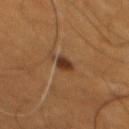Findings:
- workup: no biopsy performed (imaged during a skin exam)
- diameter: about 2.5 mm
- image source: ~15 mm tile from a whole-body skin photo
- patient: male, in their 60s
- site: the mid back
- automated lesion analysis: an area of roughly 3.5 mm², a shape eccentricity near 0.8, and two-axis asymmetry of about 0.15; a lesion color around L≈34 a*≈19 b*≈30 in CIELAB and a normalized lesion–skin contrast near 10; a classifier nevus-likeness of about 100/100